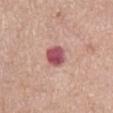Impression:
The lesion was tiled from a total-body skin photograph and was not biopsied.
Image and clinical context:
Longest diameter approximately 3 mm. Cropped from a total-body skin-imaging series; the visible field is about 15 mm. This is a white-light tile. A female subject, aged 73–77. Located on the front of the torso.